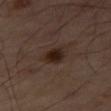The lesion was tiled from a total-body skin photograph and was not biopsied. This image is a 15 mm lesion crop taken from a total-body photograph. From the right thigh.